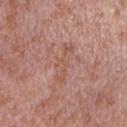The lesion was tiled from a total-body skin photograph and was not biopsied.
This is a white-light tile.
The total-body-photography lesion software estimated a lesion area of about 6 mm².
A male patient aged approximately 40.
This image is a 15 mm lesion crop taken from a total-body photograph.
The lesion's longest dimension is about 5 mm.
The lesion is on the right upper arm.